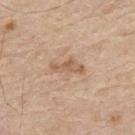biopsy status — total-body-photography surveillance lesion; no biopsy
location — the mid back
image source — total-body-photography crop, ~15 mm field of view
patient — male, in their 80s